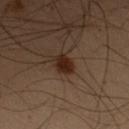Q: Was this lesion biopsied?
A: total-body-photography surveillance lesion; no biopsy
Q: What did automated image analysis measure?
A: a footprint of about 4 mm², an eccentricity of roughly 0.8, and two-axis asymmetry of about 0.25; a classifier nevus-likeness of about 95/100 and a lesion-detection confidence of about 100/100
Q: What is the anatomic site?
A: the left forearm
Q: Who is the patient?
A: female, about 50 years old
Q: What kind of image is this?
A: ~15 mm crop, total-body skin-cancer survey
Q: Illumination type?
A: cross-polarized illumination
Q: What is the lesion's diameter?
A: about 3 mm